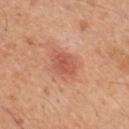Findings:
- notes: imaged on a skin check; not biopsied
- illumination: white-light
- lesion size: ≈3 mm
- image: ~15 mm tile from a whole-body skin photo
- image-analysis metrics: a lesion area of about 5.5 mm², a shape eccentricity near 0.6, and a shape-asymmetry score of about 0.25 (0 = symmetric); about 9 CIELAB-L* units darker than the surrounding skin and a normalized border contrast of about 6; a classifier nevus-likeness of about 90/100 and a lesion-detection confidence of about 100/100
- site: the mid back
- patient: male, aged around 45